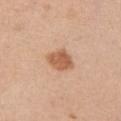<tbp_lesion>
  <biopsy_status>not biopsied; imaged during a skin examination</biopsy_status>
  <lesion_size>
    <long_diameter_mm_approx>3.5</long_diameter_mm_approx>
  </lesion_size>
  <site>right upper arm</site>
  <automated_metrics>
    <area_mm2_approx>7.0</area_mm2_approx>
    <eccentricity>0.65</eccentricity>
    <shape_asymmetry>0.2</shape_asymmetry>
    <cielab_L>59</cielab_L>
    <cielab_a>23</cielab_a>
    <cielab_b>34</cielab_b>
    <vs_skin_darker_L>12.0</vs_skin_darker_L>
    <vs_skin_contrast_norm>8.0</vs_skin_contrast_norm>
  </automated_metrics>
  <patient>
    <sex>female</sex>
    <age_approx>30</age_approx>
  </patient>
  <image>
    <source>total-body photography crop</source>
    <field_of_view_mm>15</field_of_view_mm>
  </image>
</tbp_lesion>